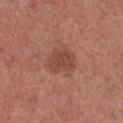The lesion was tiled from a total-body skin photograph and was not biopsied.
The subject is a female aged 53 to 57.
This is a white-light tile.
A close-up tile cropped from a whole-body skin photograph, about 15 mm across.
Automated tile analysis of the lesion measured a mean CIELAB color near L≈46 a*≈25 b*≈28 and a lesion–skin lightness drop of about 8. It also reported a nevus-likeness score of about 15/100.
Located on the front of the torso.